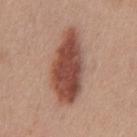– workup · imaged on a skin check; not biopsied
– anatomic site · the front of the torso
– acquisition · ~15 mm tile from a whole-body skin photo
– tile lighting · white-light
– lesion diameter · ~9 mm (longest diameter)
– patient · male, about 30 years old